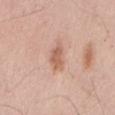biopsy status: imaged on a skin check; not biopsied | subject: male, approximately 55 years of age | anatomic site: the back | image source: ~15 mm tile from a whole-body skin photo.